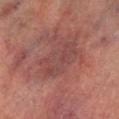Findings:
- notes · imaged on a skin check; not biopsied
- site · the leg
- subject · male, aged approximately 75
- tile lighting · cross-polarized
- TBP lesion metrics · a mean CIELAB color near L≈36 a*≈20 b*≈18, roughly 5 lightness units darker than nearby skin, and a lesion-to-skin contrast of about 4.5 (normalized; higher = more distinct); border irregularity of about 4.5 on a 0–10 scale, internal color variation of about 3 on a 0–10 scale, and radial color variation of about 1; a nevus-likeness score of about 0/100 and lesion-presence confidence of about 85/100
- lesion diameter · about 5.5 mm
- acquisition · ~15 mm crop, total-body skin-cancer survey